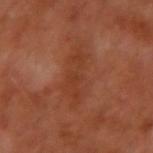<lesion>
<biopsy_status>not biopsied; imaged during a skin examination</biopsy_status>
<site>left arm</site>
<lesion_size>
  <long_diameter_mm_approx>6.5</long_diameter_mm_approx>
</lesion_size>
<patient>
  <sex>male</sex>
  <age_approx>50</age_approx>
</patient>
<image>
  <source>total-body photography crop</source>
  <field_of_view_mm>15</field_of_view_mm>
</image>
</lesion>